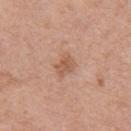The lesion was tiled from a total-body skin photograph and was not biopsied. The patient is a female roughly 55 years of age. On the left upper arm. A 15 mm close-up extracted from a 3D total-body photography capture.